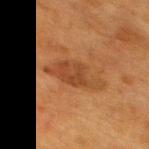Case summary:
• biopsy status — no biopsy performed (imaged during a skin exam)
• diameter — ~6.5 mm (longest diameter)
• image source — ~15 mm crop, total-body skin-cancer survey
• lighting — cross-polarized illumination
• body site — the upper back
• subject — female, aged 53–57